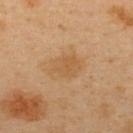Recorded during total-body skin imaging; not selected for excision or biopsy. Measured at roughly 4 mm in maximum diameter. The total-body-photography lesion software estimated a mean CIELAB color near L≈57 a*≈19 b*≈39 and a lesion-to-skin contrast of about 5.5 (normalized; higher = more distinct). A 15 mm close-up extracted from a 3D total-body photography capture. The lesion is on the upper back. A male subject, about 40 years old.